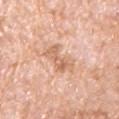workup = no biopsy performed (imaged during a skin exam)
site = the left upper arm
subject = male, approximately 80 years of age
lesion size = about 4 mm
imaging modality = total-body-photography crop, ~15 mm field of view